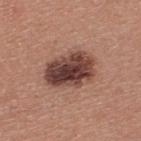Part of a total-body skin-imaging series; this lesion was reviewed on a skin check and was not flagged for biopsy. This is a white-light tile. Measured at roughly 5.5 mm in maximum diameter. The patient is a male in their 40s. This image is a 15 mm lesion crop taken from a total-body photograph. On the upper back.